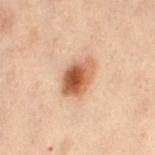notes: total-body-photography surveillance lesion; no biopsy
illumination: cross-polarized illumination
body site: the abdomen
patient: female, aged around 55
image: total-body-photography crop, ~15 mm field of view